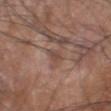{"image": {"source": "total-body photography crop", "field_of_view_mm": 15}, "automated_metrics": {"nevus_likeness_0_100": 0, "lesion_detection_confidence_0_100": 75}, "lighting": "white-light", "lesion_size": {"long_diameter_mm_approx": 2.5}, "site": "head or neck", "patient": {"sex": "male", "age_approx": 60}}